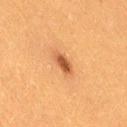<case>
<biopsy_status>not biopsied; imaged during a skin examination</biopsy_status>
<site>right thigh</site>
<lesion_size>
  <long_diameter_mm_approx>3.0</long_diameter_mm_approx>
</lesion_size>
<patient>
  <sex>female</sex>
  <age_approx>40</age_approx>
</patient>
<image>
  <source>total-body photography crop</source>
  <field_of_view_mm>15</field_of_view_mm>
</image>
<lighting>cross-polarized</lighting>
</case>